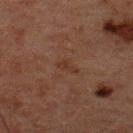Impression: Recorded during total-body skin imaging; not selected for excision or biopsy. Acquisition and patient details: Approximately 2.5 mm at its widest. A male subject aged approximately 50. A close-up tile cropped from a whole-body skin photograph, about 15 mm across. Automated tile analysis of the lesion measured a mean CIELAB color near L≈26 a*≈16 b*≈22, about 4 CIELAB-L* units darker than the surrounding skin, and a normalized border contrast of about 5.5. The software also gave a nevus-likeness score of about 0/100 and a lesion-detection confidence of about 100/100. Located on the upper back.